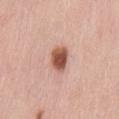| feature | finding |
|---|---|
| biopsy status | total-body-photography surveillance lesion; no biopsy |
| illumination | white-light illumination |
| site | the lower back |
| automated lesion analysis | a mean CIELAB color near L≈54 a*≈25 b*≈29, about 17 CIELAB-L* units darker than the surrounding skin, and a normalized border contrast of about 11.5; a peripheral color-asymmetry measure near 1 |
| imaging modality | ~15 mm tile from a whole-body skin photo |
| subject | female, about 45 years old |